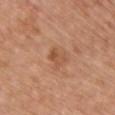follow-up = no biopsy performed (imaged during a skin exam); subject = female, aged around 60; image = total-body-photography crop, ~15 mm field of view; location = the chest.